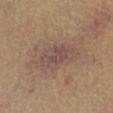TBP lesion metrics: a mean CIELAB color near L≈49 a*≈16 b*≈23, about 7 CIELAB-L* units darker than the surrounding skin, and a lesion-to-skin contrast of about 6.5 (normalized; higher = more distinct); border irregularity of about 3 on a 0–10 scale | diameter: ~6 mm (longest diameter) | patient: male, about 70 years old | image source: ~15 mm crop, total-body skin-cancer survey | anatomic site: the front of the torso.